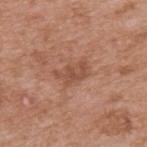{"image": {"source": "total-body photography crop", "field_of_view_mm": 15}, "lighting": "white-light", "site": "upper back", "patient": {"sex": "male", "age_approx": 55}}